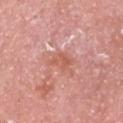follow-up = imaged on a skin check; not biopsied
imaging modality = total-body-photography crop, ~15 mm field of view
patient = male, aged 68 to 72
lesion diameter = ≈3.5 mm
automated metrics = a mean CIELAB color near L≈59 a*≈27 b*≈29, about 8 CIELAB-L* units darker than the surrounding skin, and a normalized border contrast of about 6
location = the head or neck